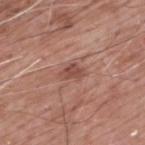Q: Was a biopsy performed?
A: catalogued during a skin exam; not biopsied
Q: What kind of image is this?
A: ~15 mm crop, total-body skin-cancer survey
Q: What are the patient's age and sex?
A: male, aged 58–62
Q: What is the anatomic site?
A: the upper back
Q: Lesion size?
A: ~2.5 mm (longest diameter)